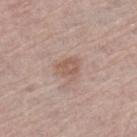The lesion was tiled from a total-body skin photograph and was not biopsied. Approximately 3 mm at its widest. Cropped from a whole-body photographic skin survey; the tile spans about 15 mm. The subject is a male aged 73–77. Located on the left thigh.